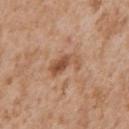follow-up=imaged on a skin check; not biopsied | lesion size=~4 mm (longest diameter) | acquisition=~15 mm crop, total-body skin-cancer survey | site=the chest | subject=male, about 65 years old | illumination=white-light.